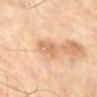Impression: The lesion was tiled from a total-body skin photograph and was not biopsied. Background: A male patient, aged 63 to 67. Captured under cross-polarized illumination. The lesion is on the right lower leg. An algorithmic analysis of the crop reported a footprint of about 5 mm² and a shape-asymmetry score of about 0.35 (0 = symmetric). And it measured a border-irregularity index near 3.5/10, a within-lesion color-variation index near 3.5/10, and radial color variation of about 1.5. And it measured a nevus-likeness score of about 0/100 and a lesion-detection confidence of about 100/100. A 15 mm crop from a total-body photograph taken for skin-cancer surveillance.